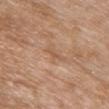Clinical impression:
The lesion was photographed on a routine skin check and not biopsied; there is no pathology result.
Image and clinical context:
A female subject, in their 60s. The lesion is located on the chest. Imaged with white-light lighting. A close-up tile cropped from a whole-body skin photograph, about 15 mm across.